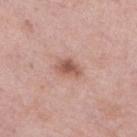No biopsy was performed on this lesion — it was imaged during a full skin examination and was not determined to be concerning. Cropped from a total-body skin-imaging series; the visible field is about 15 mm. A female subject roughly 55 years of age. Captured under white-light illumination. Located on the right lower leg.